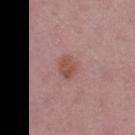{"biopsy_status": "not biopsied; imaged during a skin examination", "patient": {"sex": "female", "age_approx": 45}, "image": {"source": "total-body photography crop", "field_of_view_mm": 15}, "lesion_size": {"long_diameter_mm_approx": 3.0}, "site": "left thigh"}